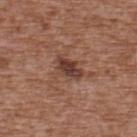| key | value |
|---|---|
| follow-up | catalogued during a skin exam; not biopsied |
| lighting | white-light illumination |
| image | ~15 mm crop, total-body skin-cancer survey |
| lesion diameter | about 3.5 mm |
| location | the back |
| subject | male, about 75 years old |